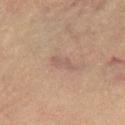Case summary:
• workup · total-body-photography surveillance lesion; no biopsy
• location · the left leg
• acquisition · ~15 mm tile from a whole-body skin photo
• lighting · cross-polarized illumination
• subject · female, roughly 65 years of age
• diameter · ≈3 mm